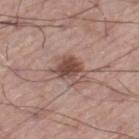Imaged during a routine full-body skin examination; the lesion was not biopsied and no histopathology is available.
A male patient, aged 58 to 62.
The total-body-photography lesion software estimated two-axis asymmetry of about 0.35. And it measured a lesion-to-skin contrast of about 9 (normalized; higher = more distinct). The analysis additionally found a border-irregularity index near 4/10 and a peripheral color-asymmetry measure near 2.
This is a white-light tile.
Cropped from a whole-body photographic skin survey; the tile spans about 15 mm.
The lesion is located on the left thigh.